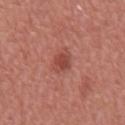Q: Was a biopsy performed?
A: catalogued during a skin exam; not biopsied
Q: What is the anatomic site?
A: the mid back
Q: Who is the patient?
A: male, aged 63 to 67
Q: How was the tile lit?
A: white-light illumination
Q: Lesion size?
A: about 2.5 mm
Q: How was this image acquired?
A: ~15 mm tile from a whole-body skin photo